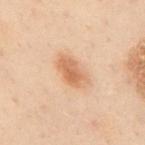Imaged with cross-polarized lighting.
A close-up tile cropped from a whole-body skin photograph, about 15 mm across.
The patient is a male in their 50s.
Approximately 4 mm at its widest.
Located on the upper back.